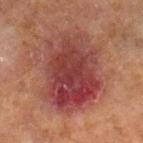  image:
    source: total-body photography crop
    field_of_view_mm: 15
  lighting: cross-polarized
  patient:
    sex: male
    age_approx: 65
  site: left lower leg